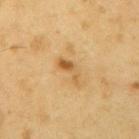| field | value |
|---|---|
| follow-up | catalogued during a skin exam; not biopsied |
| subject | male, aged around 60 |
| acquisition | 15 mm crop, total-body photography |
| tile lighting | cross-polarized illumination |
| automated lesion analysis | a footprint of about 4 mm², an outline eccentricity of about 0.9 (0 = round, 1 = elongated), and two-axis asymmetry of about 0.45; a mean CIELAB color near L≈58 a*≈19 b*≈44 and roughly 10 lightness units darker than nearby skin; border irregularity of about 5.5 on a 0–10 scale, a within-lesion color-variation index near 2.5/10, and peripheral color asymmetry of about 0.5 |
| body site | the arm |
| lesion size | ≈3.5 mm |